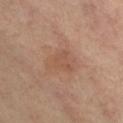No biopsy was performed on this lesion — it was imaged during a full skin examination and was not determined to be concerning. A female patient roughly 50 years of age. The lesion's longest dimension is about 3 mm. This is a cross-polarized tile. A 15 mm crop from a total-body photograph taken for skin-cancer surveillance. On the leg. An algorithmic analysis of the crop reported about 6 CIELAB-L* units darker than the surrounding skin and a normalized lesion–skin contrast near 4.5. The analysis additionally found a border-irregularity rating of about 4/10 and peripheral color asymmetry of about 0.5.